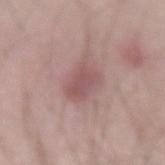This lesion was catalogued during total-body skin photography and was not selected for biopsy.
From the left forearm.
Approximately 3.5 mm at its widest.
A male subject roughly 35 years of age.
A region of skin cropped from a whole-body photographic capture, roughly 15 mm wide.
This is a white-light tile.